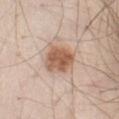Notes:
– notes · catalogued during a skin exam; not biopsied
– site · the abdomen
– TBP lesion metrics · an area of roughly 12 mm², an outline eccentricity of about 0.35 (0 = round, 1 = elongated), and a symmetry-axis asymmetry near 0.15
– patient · male, aged approximately 40
– image · total-body-photography crop, ~15 mm field of view
– tile lighting · white-light
– lesion size · ~4 mm (longest diameter)